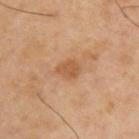Impression:
The lesion was tiled from a total-body skin photograph and was not biopsied.
Context:
Measured at roughly 2.5 mm in maximum diameter. The tile uses cross-polarized illumination. The patient is a male roughly 40 years of age. From the back. A 15 mm close-up extracted from a 3D total-body photography capture.